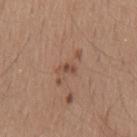This lesion was catalogued during total-body skin photography and was not selected for biopsy.
From the mid back.
Automated tile analysis of the lesion measured a footprint of about 2 mm², an outline eccentricity of about 0.85 (0 = round, 1 = elongated), and a shape-asymmetry score of about 0.3 (0 = symmetric). And it measured a mean CIELAB color near L≈48 a*≈20 b*≈27 and a normalized lesion–skin contrast near 6.5. It also reported border irregularity of about 2.5 on a 0–10 scale and a color-variation rating of about 0/10. The software also gave a nevus-likeness score of about 5/100.
The patient is a male aged 23 to 27.
Imaged with white-light lighting.
A close-up tile cropped from a whole-body skin photograph, about 15 mm across.